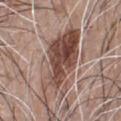{"biopsy_status": "not biopsied; imaged during a skin examination", "automated_metrics": {"cielab_L": 47, "cielab_a": 19, "cielab_b": 24, "vs_skin_darker_L": 14.0, "vs_skin_contrast_norm": 10.5, "nevus_likeness_0_100": 100, "lesion_detection_confidence_0_100": 95}, "patient": {"sex": "male", "age_approx": 45}, "site": "chest", "image": {"source": "total-body photography crop", "field_of_view_mm": 15}}